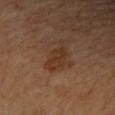No biopsy was performed on this lesion — it was imaged during a full skin examination and was not determined to be concerning. A female subject aged 53 to 57. An algorithmic analysis of the crop reported a mean CIELAB color near L≈28 a*≈17 b*≈25 and a normalized border contrast of about 6.5. The tile uses cross-polarized illumination. A 15 mm close-up tile from a total-body photography series done for melanoma screening. Measured at roughly 4.5 mm in maximum diameter. The lesion is on the right forearm.